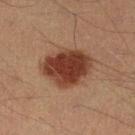Impression:
Part of a total-body skin-imaging series; this lesion was reviewed on a skin check and was not flagged for biopsy.
Image and clinical context:
A 15 mm close-up tile from a total-body photography series done for melanoma screening. Approximately 6 mm at its widest. The total-body-photography lesion software estimated an eccentricity of roughly 0.65 and two-axis asymmetry of about 0.2. And it measured an average lesion color of about L≈35 a*≈21 b*≈27 (CIELAB) and roughly 14 lightness units darker than nearby skin. On the left lower leg. This is a cross-polarized tile. A male subject aged 38 to 42.